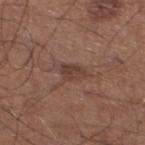biopsy_status: not biopsied; imaged during a skin examination
patient:
  sex: male
  age_approx: 60
site: left lower leg
image:
  source: total-body photography crop
  field_of_view_mm: 15